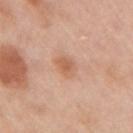Q: Is there a histopathology result?
A: no biopsy performed (imaged during a skin exam)
Q: What is the anatomic site?
A: the right upper arm
Q: What kind of image is this?
A: 15 mm crop, total-body photography
Q: What lighting was used for the tile?
A: white-light illumination
Q: Patient demographics?
A: female, in their mid-40s
Q: Automated lesion metrics?
A: a mean CIELAB color near L≈61 a*≈23 b*≈33, a lesion–skin lightness drop of about 9, and a normalized border contrast of about 6; a border-irregularity index near 1.5/10, a color-variation rating of about 2.5/10, and a peripheral color-asymmetry measure near 1; lesion-presence confidence of about 100/100
Q: How large is the lesion?
A: ≈2.5 mm